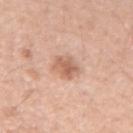workup: total-body-photography surveillance lesion; no biopsy
lighting: white-light
diameter: ~3.5 mm (longest diameter)
acquisition: ~15 mm tile from a whole-body skin photo
patient: female, approximately 40 years of age
location: the left forearm